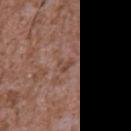Q: Was this lesion biopsied?
A: no biopsy performed (imaged during a skin exam)
Q: Automated lesion metrics?
A: a lesion color around L≈46 a*≈19 b*≈26 in CIELAB, about 6 CIELAB-L* units darker than the surrounding skin, and a normalized border contrast of about 5; a border-irregularity rating of about 3.5/10 and internal color variation of about 3.5 on a 0–10 scale
Q: Where on the body is the lesion?
A: the chest
Q: How large is the lesion?
A: about 2.5 mm
Q: How was the tile lit?
A: white-light illumination
Q: What is the imaging modality?
A: ~15 mm tile from a whole-body skin photo
Q: What are the patient's age and sex?
A: male, about 45 years old